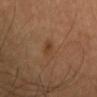Findings:
• workup · no biopsy performed (imaged during a skin exam)
• subject · male, aged 53–57
• lesion diameter · ≈2.5 mm
• site · the head or neck
• imaging modality · 15 mm crop, total-body photography